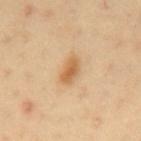workup: catalogued during a skin exam; not biopsied | patient: male, approximately 40 years of age | lesion diameter: ~3.5 mm (longest diameter) | image: 15 mm crop, total-body photography | anatomic site: the mid back.